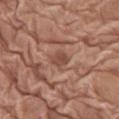{"biopsy_status": "not biopsied; imaged during a skin examination", "patient": {"sex": "female", "age_approx": 75}, "automated_metrics": {"cielab_L": 48, "cielab_a": 22, "cielab_b": 29, "vs_skin_darker_L": 8.0, "vs_skin_contrast_norm": 6.5, "border_irregularity_0_10": 1.5, "color_variation_0_10": 1.5, "peripheral_color_asymmetry": 0.5, "nevus_likeness_0_100": 0}, "lighting": "white-light", "site": "leg", "image": {"source": "total-body photography crop", "field_of_view_mm": 15}, "lesion_size": {"long_diameter_mm_approx": 2.5}}